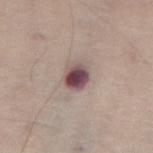Captured during whole-body skin photography for melanoma surveillance; the lesion was not biopsied.
The lesion's longest dimension is about 3 mm.
A male patient, aged approximately 75.
Captured under white-light illumination.
A region of skin cropped from a whole-body photographic capture, roughly 15 mm wide.
From the abdomen.
Automated image analysis of the tile measured a lesion color around L≈47 a*≈19 b*≈16 in CIELAB and a normalized lesion–skin contrast near 12.5. And it measured an automated nevus-likeness rating near 5 out of 100 and a lesion-detection confidence of about 100/100.